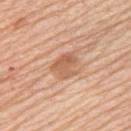- follow-up: catalogued during a skin exam; not biopsied
- size: ≈3.5 mm
- illumination: white-light illumination
- TBP lesion metrics: a lesion color around L≈61 a*≈22 b*≈34 in CIELAB and a lesion-to-skin contrast of about 6.5 (normalized; higher = more distinct); a border-irregularity rating of about 4/10 and a color-variation rating of about 3.5/10; a nevus-likeness score of about 30/100 and lesion-presence confidence of about 100/100
- imaging modality: total-body-photography crop, ~15 mm field of view
- site: the left upper arm
- patient: female, aged 63–67